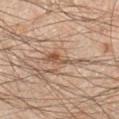This lesion was catalogued during total-body skin photography and was not selected for biopsy.
A 15 mm close-up extracted from a 3D total-body photography capture.
A male subject roughly 45 years of age.
Automated tile analysis of the lesion measured a nevus-likeness score of about 5/100 and a detector confidence of about 90 out of 100 that the crop contains a lesion.
The lesion is located on the left forearm.
Approximately 4.5 mm at its widest.